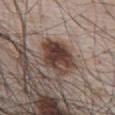No biopsy was performed on this lesion — it was imaged during a full skin examination and was not determined to be concerning.
A lesion tile, about 15 mm wide, cut from a 3D total-body photograph.
The tile uses white-light illumination.
A male subject aged 63–67.
From the abdomen.
Approximately 5 mm at its widest.